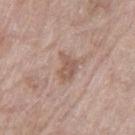The lesion was photographed on a routine skin check and not biopsied; there is no pathology result.
Automated image analysis of the tile measured a lesion area of about 5.5 mm² and an outline eccentricity of about 0.65 (0 = round, 1 = elongated). And it measured border irregularity of about 3 on a 0–10 scale and a color-variation rating of about 2/10.
Located on the left thigh.
The patient is a female aged 73–77.
Cropped from a whole-body photographic skin survey; the tile spans about 15 mm.